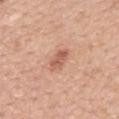biopsy_status: not biopsied; imaged during a skin examination
site: upper back
patient:
  sex: female
  age_approx: 60
image:
  source: total-body photography crop
  field_of_view_mm: 15
lighting: white-light
lesion_size:
  long_diameter_mm_approx: 3.0